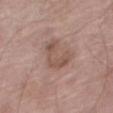{
  "biopsy_status": "not biopsied; imaged during a skin examination",
  "site": "right thigh",
  "lighting": "white-light",
  "patient": {
    "sex": "female",
    "age_approx": 75
  },
  "image": {
    "source": "total-body photography crop",
    "field_of_view_mm": 15
  },
  "automated_metrics": {
    "border_irregularity_0_10": 8.0,
    "color_variation_0_10": 3.0,
    "peripheral_color_asymmetry": 1.0,
    "nevus_likeness_0_100": 10
  },
  "lesion_size": {
    "long_diameter_mm_approx": 4.0
  }
}